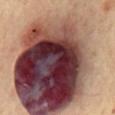Captured during whole-body skin photography for melanoma surveillance; the lesion was not biopsied. An algorithmic analysis of the crop reported a footprint of about 155 mm², a shape eccentricity near 0.8, and two-axis asymmetry of about 0.25. The software also gave a nevus-likeness score of about 0/100. A male patient approximately 70 years of age. This is a cross-polarized tile. This image is a 15 mm lesion crop taken from a total-body photograph. The lesion is on the chest. Longest diameter approximately 19.5 mm.